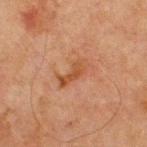notes = catalogued during a skin exam; not biopsied | automated lesion analysis = an eccentricity of roughly 0.9; a lesion color around L≈41 a*≈21 b*≈31 in CIELAB and roughly 7 lightness units darker than nearby skin; an automated nevus-likeness rating near 5 out of 100 and a lesion-detection confidence of about 100/100 | anatomic site = the chest | illumination = cross-polarized | imaging modality = ~15 mm tile from a whole-body skin photo | patient = male, aged 63 to 67 | size = ~4 mm (longest diameter).